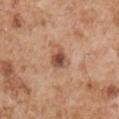This lesion was catalogued during total-body skin photography and was not selected for biopsy. A 15 mm crop from a total-body photograph taken for skin-cancer surveillance. Measured at roughly 2.5 mm in maximum diameter. Imaged with white-light lighting. The patient is a male roughly 55 years of age. Located on the right upper arm.